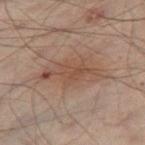The lesion was tiled from a total-body skin photograph and was not biopsied.
The lesion is located on the left leg.
A male subject aged approximately 50.
Cropped from a total-body skin-imaging series; the visible field is about 15 mm.